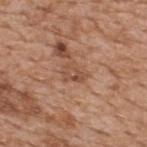The lesion was photographed on a routine skin check and not biopsied; there is no pathology result.
Located on the upper back.
The patient is a male approximately 65 years of age.
This is a white-light tile.
A 15 mm close-up extracted from a 3D total-body photography capture.
The recorded lesion diameter is about 2.5 mm.